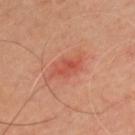Assessment:
No biopsy was performed on this lesion — it was imaged during a full skin examination and was not determined to be concerning.
Image and clinical context:
An algorithmic analysis of the crop reported a footprint of about 5.5 mm², an eccentricity of roughly 0.85, and a shape-asymmetry score of about 0.25 (0 = symmetric). And it measured a lesion color around L≈49 a*≈31 b*≈32 in CIELAB, about 8 CIELAB-L* units darker than the surrounding skin, and a normalized lesion–skin contrast near 6. From the chest. A lesion tile, about 15 mm wide, cut from a 3D total-body photograph. This is a cross-polarized tile. About 3.5 mm across. The patient is a male aged around 45.